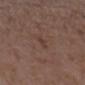No biopsy was performed on this lesion — it was imaged during a full skin examination and was not determined to be concerning.
The lesion's longest dimension is about 3 mm.
The lesion is on the left forearm.
Cropped from a total-body skin-imaging series; the visible field is about 15 mm.
The tile uses white-light illumination.
A female subject, in their mid-30s.
The lesion-visualizer software estimated a footprint of about 2.5 mm² and a symmetry-axis asymmetry near 0.3. It also reported roughly 5 lightness units darker than nearby skin. The software also gave a border-irregularity index near 3.5/10, internal color variation of about 0.5 on a 0–10 scale, and radial color variation of about 0.